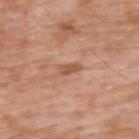| feature | finding |
|---|---|
| biopsy status | catalogued during a skin exam; not biopsied |
| lighting | white-light |
| image source | total-body-photography crop, ~15 mm field of view |
| body site | the upper back |
| subject | male, approximately 60 years of age |